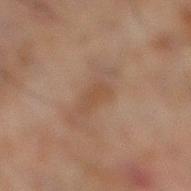Clinical impression: No biopsy was performed on this lesion — it was imaged during a full skin examination and was not determined to be concerning. Clinical summary: About 3.5 mm across. A male subject, about 45 years old. A close-up tile cropped from a whole-body skin photograph, about 15 mm across. The tile uses cross-polarized illumination. The lesion is on the leg. The lesion-visualizer software estimated a mean CIELAB color near L≈38 a*≈15 b*≈25.